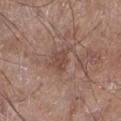No biopsy was performed on this lesion — it was imaged during a full skin examination and was not determined to be concerning. An algorithmic analysis of the crop reported a mean CIELAB color near L≈46 a*≈19 b*≈26, a lesion–skin lightness drop of about 8, and a normalized lesion–skin contrast near 6. It also reported an automated nevus-likeness rating near 0 out of 100. A male patient, aged approximately 60. Approximately 3 mm at its widest. A 15 mm close-up extracted from a 3D total-body photography capture. From the left lower leg.